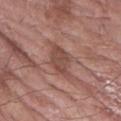{
  "biopsy_status": "not biopsied; imaged during a skin examination",
  "patient": {
    "sex": "male",
    "age_approx": 60
  },
  "site": "right forearm",
  "image": {
    "source": "total-body photography crop",
    "field_of_view_mm": 15
  }
}